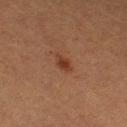workup = no biopsy performed (imaged during a skin exam) | image source = ~15 mm tile from a whole-body skin photo | lighting = cross-polarized | lesion diameter = ~3 mm (longest diameter) | subject = female, in their mid-50s | site = the left thigh.